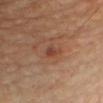workup: total-body-photography surveillance lesion; no biopsy | location: the chest | acquisition: ~15 mm tile from a whole-body skin photo | lesion diameter: ≈1.5 mm | automated lesion analysis: an eccentricity of roughly 0.65 and a symmetry-axis asymmetry near 0.3; a lesion color around L≈41 a*≈23 b*≈28 in CIELAB and a lesion-to-skin contrast of about 7 (normalized; higher = more distinct); a border-irregularity rating of about 2.5/10 and internal color variation of about 0 on a 0–10 scale; a nevus-likeness score of about 5/100 and a lesion-detection confidence of about 100/100 | patient: male, aged 63–67.